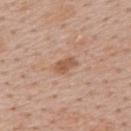Notes:
– biopsy status: catalogued during a skin exam; not biopsied
– lesion diameter: ~2.5 mm (longest diameter)
– image-analysis metrics: a footprint of about 3.5 mm² and an eccentricity of roughly 0.85; a lesion color around L≈55 a*≈22 b*≈32 in CIELAB and a lesion-to-skin contrast of about 7.5 (normalized; higher = more distinct); an automated nevus-likeness rating near 35 out of 100 and a detector confidence of about 100 out of 100 that the crop contains a lesion
– body site: the upper back
– subject: male, roughly 55 years of age
– image source: ~15 mm crop, total-body skin-cancer survey
– lighting: white-light